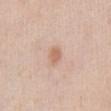No biopsy was performed on this lesion — it was imaged during a full skin examination and was not determined to be concerning.
Automated image analysis of the tile measured a border-irregularity index near 1.5/10. The analysis additionally found a classifier nevus-likeness of about 60/100 and a lesion-detection confidence of about 100/100.
A male patient, aged 38 to 42.
About 2 mm across.
Located on the abdomen.
A region of skin cropped from a whole-body photographic capture, roughly 15 mm wide.